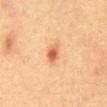The lesion is located on the abdomen.
A male subject aged around 40.
Automated image analysis of the tile measured a footprint of about 4 mm² and an eccentricity of roughly 0.8. It also reported border irregularity of about 2 on a 0–10 scale, a within-lesion color-variation index near 3/10, and radial color variation of about 1. The software also gave a classifier nevus-likeness of about 90/100.
Approximately 3 mm at its widest.
Cropped from a total-body skin-imaging series; the visible field is about 15 mm.
Imaged with cross-polarized lighting.